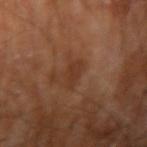notes = total-body-photography surveillance lesion; no biopsy | body site = the right upper arm | subject = male, aged 68 to 72 | image = ~15 mm tile from a whole-body skin photo.